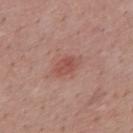<lesion>
<biopsy_status>not biopsied; imaged during a skin examination</biopsy_status>
<lighting>white-light</lighting>
<lesion_size>
  <long_diameter_mm_approx>3.5</long_diameter_mm_approx>
</lesion_size>
<image>
  <source>total-body photography crop</source>
  <field_of_view_mm>15</field_of_view_mm>
</image>
<site>upper back</site>
<patient>
  <sex>male</sex>
  <age_approx>25</age_approx>
</patient>
<automated_metrics>
  <area_mm2_approx>5.5</area_mm2_approx>
  <eccentricity>0.8</eccentricity>
  <shape_asymmetry>0.25</shape_asymmetry>
  <border_irregularity_0_10>2.5</border_irregularity_0_10>
  <color_variation_0_10>3.0</color_variation_0_10>
  <peripheral_color_asymmetry>1.0</peripheral_color_asymmetry>
</automated_metrics>
</lesion>